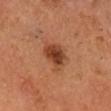Q: Was a biopsy performed?
A: catalogued during a skin exam; not biopsied
Q: What kind of image is this?
A: ~15 mm tile from a whole-body skin photo
Q: Patient demographics?
A: male, aged approximately 65
Q: Lesion location?
A: the head or neck
Q: How large is the lesion?
A: ≈4.5 mm
Q: What lighting was used for the tile?
A: cross-polarized illumination
Q: Automated lesion metrics?
A: an area of roughly 10 mm², a shape eccentricity near 0.65, and a shape-asymmetry score of about 0.3 (0 = symmetric); a lesion color around L≈41 a*≈24 b*≈32 in CIELAB, about 11 CIELAB-L* units darker than the surrounding skin, and a lesion-to-skin contrast of about 8.5 (normalized; higher = more distinct); a within-lesion color-variation index near 4.5/10; a nevus-likeness score of about 95/100 and a detector confidence of about 100 out of 100 that the crop contains a lesion